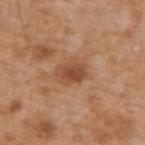This lesion was catalogued during total-body skin photography and was not selected for biopsy. The lesion's longest dimension is about 3.5 mm. On the upper back. A lesion tile, about 15 mm wide, cut from a 3D total-body photograph. A male subject aged around 65.